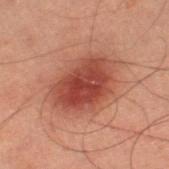biopsy status — imaged on a skin check; not biopsied
automated metrics — an area of roughly 22 mm², an outline eccentricity of about 0.7 (0 = round, 1 = elongated), and a shape-asymmetry score of about 0.15 (0 = symmetric); an average lesion color of about L≈35 a*≈24 b*≈24 (CIELAB), a lesion–skin lightness drop of about 10, and a normalized lesion–skin contrast near 9; a border-irregularity index near 2/10 and radial color variation of about 1
image source — total-body-photography crop, ~15 mm field of view
subject — male, aged 58–62
tile lighting — cross-polarized
site — the left thigh
size — ≈6 mm